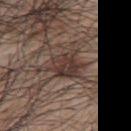The lesion was photographed on a routine skin check and not biopsied; there is no pathology result.
Captured under white-light illumination.
The lesion is located on the upper back.
A male patient, in their 70s.
A close-up tile cropped from a whole-body skin photograph, about 15 mm across.